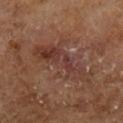Part of a total-body skin-imaging series; this lesion was reviewed on a skin check and was not flagged for biopsy.
Cropped from a total-body skin-imaging series; the visible field is about 15 mm.
About 8 mm across.
A male subject approximately 65 years of age.
The tile uses cross-polarized illumination.